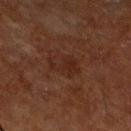Clinical impression:
The lesion was photographed on a routine skin check and not biopsied; there is no pathology result.
Clinical summary:
The subject is a male aged 73–77. The lesion-visualizer software estimated a lesion area of about 6 mm², a shape eccentricity near 0.75, and a symmetry-axis asymmetry near 0.6. And it measured a classifier nevus-likeness of about 0/100 and a lesion-detection confidence of about 100/100. A 15 mm crop from a total-body photograph taken for skin-cancer surveillance. Longest diameter approximately 4 mm. From the right forearm.